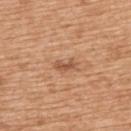Captured during whole-body skin photography for melanoma surveillance; the lesion was not biopsied.
The patient is a female roughly 55 years of age.
A lesion tile, about 15 mm wide, cut from a 3D total-body photograph.
About 2.5 mm across.
On the back.
The tile uses white-light illumination.